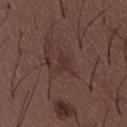Assessment:
Part of a total-body skin-imaging series; this lesion was reviewed on a skin check and was not flagged for biopsy.
Acquisition and patient details:
The tile uses white-light illumination. Measured at roughly 2.5 mm in maximum diameter. From the abdomen. A male patient, aged around 50. A 15 mm close-up extracted from a 3D total-body photography capture.